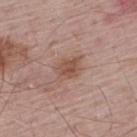<lesion>
<biopsy_status>not biopsied; imaged during a skin examination</biopsy_status>
<site>back</site>
<image>
  <source>total-body photography crop</source>
  <field_of_view_mm>15</field_of_view_mm>
</image>
<patient>
  <sex>male</sex>
  <age_approx>65</age_approx>
</patient>
</lesion>